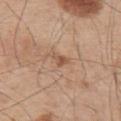Image and clinical context:
Longest diameter approximately 2.5 mm. A roughly 15 mm field-of-view crop from a total-body skin photograph. Captured under white-light illumination. The lesion is located on the upper back. A male patient, about 55 years old.